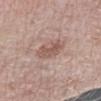| key | value |
|---|---|
| workup | total-body-photography surveillance lesion; no biopsy |
| body site | the right forearm |
| lesion diameter | about 3 mm |
| image-analysis metrics | a lesion area of about 6 mm²; a normalized lesion–skin contrast near 6.5; a border-irregularity rating of about 3.5/10 and a color-variation rating of about 3/10; a lesion-detection confidence of about 100/100 |
| image | ~15 mm tile from a whole-body skin photo |
| subject | female, roughly 65 years of age |
| lighting | white-light |